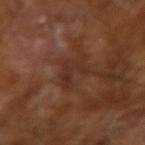biopsy_status: not biopsied; imaged during a skin examination
patient:
  sex: male
  age_approx: 65
image:
  source: total-body photography crop
  field_of_view_mm: 15
lighting: cross-polarized